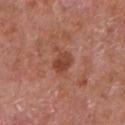No biopsy was performed on this lesion — it was imaged during a full skin examination and was not determined to be concerning. Located on the chest. A roughly 15 mm field-of-view crop from a total-body skin photograph. A male subject, aged around 65.